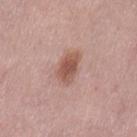biopsy status: catalogued during a skin exam; not biopsied
imaging modality: total-body-photography crop, ~15 mm field of view
body site: the left thigh
patient: female, aged approximately 35
lighting: white-light illumination
TBP lesion metrics: a lesion area of about 6.5 mm² and a shape eccentricity near 0.75; a mean CIELAB color near L≈53 a*≈22 b*≈26, a lesion–skin lightness drop of about 12, and a normalized lesion–skin contrast near 8.5; border irregularity of about 2.5 on a 0–10 scale, a color-variation rating of about 3/10, and radial color variation of about 1; a nevus-likeness score of about 90/100 and a lesion-detection confidence of about 100/100